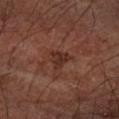Case summary:
* workup · no biopsy performed (imaged during a skin exam)
* site · the left forearm
* imaging modality · ~15 mm tile from a whole-body skin photo
* patient · male, aged 58 to 62
* automated metrics · an automated nevus-likeness rating near 0 out of 100 and a lesion-detection confidence of about 100/100